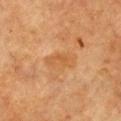  biopsy_status: not biopsied; imaged during a skin examination
  lighting: cross-polarized
  automated_metrics:
    area_mm2_approx: 5.0
    eccentricity: 0.9
    shape_asymmetry: 0.25
    cielab_L: 49
    cielab_a: 22
    cielab_b: 38
    vs_skin_darker_L: 6.0
    border_irregularity_0_10: 3.0
    color_variation_0_10: 1.5
    peripheral_color_asymmetry: 0.5
  patient:
    sex: male
    age_approx: 85+
  site: front of the torso
  image:
    source: total-body photography crop
    field_of_view_mm: 15